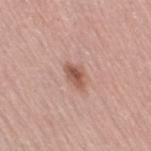Captured during whole-body skin photography for melanoma surveillance; the lesion was not biopsied. Imaged with white-light lighting. From the leg. A female subject aged around 65. Cropped from a total-body skin-imaging series; the visible field is about 15 mm. The lesion's longest dimension is about 3 mm.